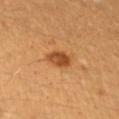Captured during whole-body skin photography for melanoma surveillance; the lesion was not biopsied. Approximately 3 mm at its widest. A female subject aged 23–27. Imaged with cross-polarized lighting. On the left forearm. A 15 mm close-up tile from a total-body photography series done for melanoma screening.